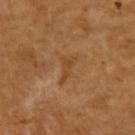Impression: The lesion was photographed on a routine skin check and not biopsied; there is no pathology result. Image and clinical context: Approximately 3.5 mm at its widest. On the arm. Cropped from a whole-body photographic skin survey; the tile spans about 15 mm. A female patient, roughly 55 years of age. Imaged with cross-polarized lighting.